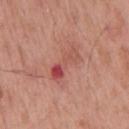Assessment: The lesion was tiled from a total-body skin photograph and was not biopsied. Acquisition and patient details: A 15 mm crop from a total-body photograph taken for skin-cancer surveillance. The patient is a male aged 53 to 57. Measured at roughly 4.5 mm in maximum diameter. This is a white-light tile. Located on the mid back.